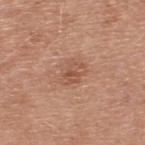Clinical impression: No biopsy was performed on this lesion — it was imaged during a full skin examination and was not determined to be concerning. Image and clinical context: A male subject, aged around 50. A roughly 15 mm field-of-view crop from a total-body skin photograph. Longest diameter approximately 3 mm. The lesion is located on the upper back.